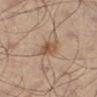Findings:
* biopsy status · imaged on a skin check; not biopsied
* illumination · cross-polarized illumination
* anatomic site · the left leg
* acquisition · ~15 mm crop, total-body skin-cancer survey
* patient · male, aged 48–52
* size · about 3 mm